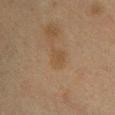Case summary:
– imaging modality · total-body-photography crop, ~15 mm field of view
– illumination · cross-polarized
– subject · female, about 40 years old
– site · the chest
– size · ≈2.5 mm